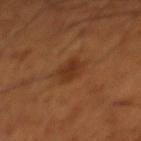Clinical impression: The lesion was photographed on a routine skin check and not biopsied; there is no pathology result. Clinical summary: The lesion is on the left lower leg. Cropped from a whole-body photographic skin survey; the tile spans about 15 mm. Approximately 3 mm at its widest. A male subject, approximately 65 years of age. The tile uses cross-polarized illumination.